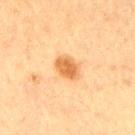Imaged during a routine full-body skin examination; the lesion was not biopsied and no histopathology is available. An algorithmic analysis of the crop reported a border-irregularity index near 2/10, a color-variation rating of about 2.5/10, and peripheral color asymmetry of about 1. The analysis additionally found an automated nevus-likeness rating near 100 out of 100 and a lesion-detection confidence of about 100/100. On the left upper arm. A male subject, aged 63–67. Measured at roughly 3.5 mm in maximum diameter. A 15 mm close-up tile from a total-body photography series done for melanoma screening. Captured under cross-polarized illumination.